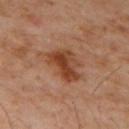Impression:
This lesion was catalogued during total-body skin photography and was not selected for biopsy.
Context:
A male patient, aged 58–62. This is a cross-polarized tile. The lesion is on the mid back. Longest diameter approximately 4.5 mm. Cropped from a total-body skin-imaging series; the visible field is about 15 mm. Automated tile analysis of the lesion measured an eccentricity of roughly 0.7 and a symmetry-axis asymmetry near 0.3. The software also gave an automated nevus-likeness rating near 80 out of 100 and a lesion-detection confidence of about 100/100.